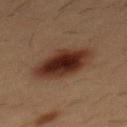Clinical impression:
The lesion was tiled from a total-body skin photograph and was not biopsied.
Clinical summary:
Longest diameter approximately 6 mm. A close-up tile cropped from a whole-body skin photograph, about 15 mm across. The lesion is on the chest. This is a cross-polarized tile. An algorithmic analysis of the crop reported a footprint of about 20 mm² and two-axis asymmetry of about 0.1. It also reported a mean CIELAB color near L≈26 a*≈18 b*≈22, roughly 12 lightness units darker than nearby skin, and a lesion-to-skin contrast of about 12.5 (normalized; higher = more distinct). The analysis additionally found border irregularity of about 1.5 on a 0–10 scale and peripheral color asymmetry of about 1.5. It also reported an automated nevus-likeness rating near 100 out of 100 and lesion-presence confidence of about 100/100. A male patient aged 53–57.